On the leg · cropped from a whole-body photographic skin survey; the tile spans about 15 mm · a female patient, aged around 25.
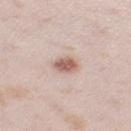Q: How was the tile lit?
A: white-light illumination
Q: How large is the lesion?
A: about 3 mm
Q: Automated lesion metrics?
A: internal color variation of about 3 on a 0–10 scale and a peripheral color-asymmetry measure near 1
Q: What is the histopathologic diagnosis?
A: a dysplastic (Clark) nevus — a benign skin lesion